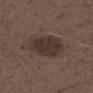Recorded during total-body skin imaging; not selected for excision or biopsy. A male patient, roughly 70 years of age. From the leg. Automated image analysis of the tile measured an automated nevus-likeness rating near 35 out of 100. A region of skin cropped from a whole-body photographic capture, roughly 15 mm wide. The recorded lesion diameter is about 5 mm.